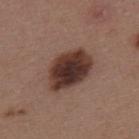Imaged during a routine full-body skin examination; the lesion was not biopsied and no histopathology is available. The recorded lesion diameter is about 6 mm. Located on the upper back. Automated tile analysis of the lesion measured a footprint of about 18 mm², a shape eccentricity near 0.75, and a symmetry-axis asymmetry near 0.15. Cropped from a total-body skin-imaging series; the visible field is about 15 mm. A female patient aged approximately 45. The tile uses white-light illumination.